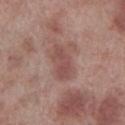Impression: This lesion was catalogued during total-body skin photography and was not selected for biopsy. Background: A 15 mm close-up extracted from a 3D total-body photography capture. Located on the right lower leg. A male subject, aged 68 to 72.